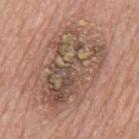Captured during whole-body skin photography for melanoma surveillance; the lesion was not biopsied. A roughly 15 mm field-of-view crop from a total-body skin photograph. The subject is a male aged approximately 60. Measured at roughly 10 mm in maximum diameter. Located on the mid back.